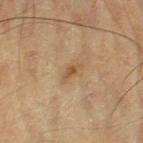Captured during whole-body skin photography for melanoma surveillance; the lesion was not biopsied.
A close-up tile cropped from a whole-body skin photograph, about 15 mm across.
A male subject, in their mid- to late 80s or older.
The lesion is on the leg.